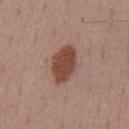The lesion was tiled from a total-body skin photograph and was not biopsied.
The lesion-visualizer software estimated a footprint of about 11 mm² and a shape eccentricity near 0.85. It also reported a lesion color around L≈45 a*≈22 b*≈26 in CIELAB and roughly 13 lightness units darker than nearby skin. And it measured a color-variation rating of about 2.5/10 and peripheral color asymmetry of about 1.
Located on the mid back.
A male patient, aged 53 to 57.
A roughly 15 mm field-of-view crop from a total-body skin photograph.
The recorded lesion diameter is about 5 mm.